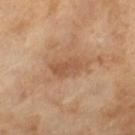follow-up: total-body-photography surveillance lesion; no biopsy | subject: male, in their mid- to late 60s | lesion size: ≈4.5 mm | image: total-body-photography crop, ~15 mm field of view | TBP lesion metrics: an outline eccentricity of about 0.85 (0 = round, 1 = elongated); a border-irregularity rating of about 3.5/10, a within-lesion color-variation index near 3/10, and peripheral color asymmetry of about 1.